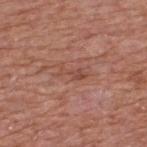follow-up = catalogued during a skin exam; not biopsied | image source = 15 mm crop, total-body photography | lighting = white-light illumination | anatomic site = the back | patient = male, about 65 years old | automated lesion analysis = a normalized border contrast of about 5.5; a color-variation rating of about 1.5/10 and a peripheral color-asymmetry measure near 0.5.